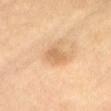Notes:
* notes · total-body-photography surveillance lesion; no biopsy
* automated lesion analysis · border irregularity of about 3 on a 0–10 scale, a color-variation rating of about 1/10, and radial color variation of about 0.5; a nevus-likeness score of about 0/100
* acquisition · ~15 mm crop, total-body skin-cancer survey
* subject · male, in their mid-60s
* size · ~2.5 mm (longest diameter)
* site · the chest
* lighting · cross-polarized illumination